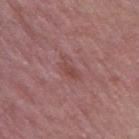Findings:
• biopsy status — imaged on a skin check; not biopsied
• site — the mid back
• size — ≈3 mm
• subject — male, aged approximately 55
• automated metrics — a lesion–skin lightness drop of about 6
• tile lighting — white-light illumination
• image source — total-body-photography crop, ~15 mm field of view